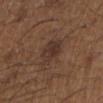workup: imaged on a skin check; not biopsied | diameter: ~3.5 mm (longest diameter) | illumination: white-light illumination | patient: male, in their 50s | acquisition: 15 mm crop, total-body photography | location: the left upper arm.